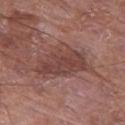{"biopsy_status": "not biopsied; imaged during a skin examination", "patient": {"sex": "male", "age_approx": 65}, "image": {"source": "total-body photography crop", "field_of_view_mm": 15}, "lesion_size": {"long_diameter_mm_approx": 7.0}, "site": "left thigh", "automated_metrics": {"area_mm2_approx": 19.0, "eccentricity": 0.8, "shape_asymmetry": 0.3, "border_irregularity_0_10": 4.5, "peripheral_color_asymmetry": 1.0, "nevus_likeness_0_100": 0}, "lighting": "white-light"}